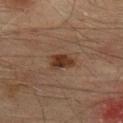{"biopsy_status": "not biopsied; imaged during a skin examination", "site": "upper back", "patient": {"sex": "male", "age_approx": 75}, "image": {"source": "total-body photography crop", "field_of_view_mm": 15}}